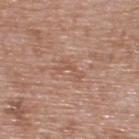Impression:
Imaged during a routine full-body skin examination; the lesion was not biopsied and no histopathology is available.
Background:
A male patient, aged around 50. The lesion is on the upper back. A 15 mm crop from a total-body photograph taken for skin-cancer surveillance. About 3.5 mm across.